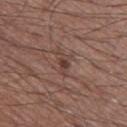This lesion was catalogued during total-body skin photography and was not selected for biopsy.
The lesion is on the leg.
Measured at roughly 3.5 mm in maximum diameter.
Automated tile analysis of the lesion measured a footprint of about 4 mm² and a symmetry-axis asymmetry near 0.4. It also reported a nevus-likeness score of about 0/100 and a detector confidence of about 100 out of 100 that the crop contains a lesion.
The patient is a male approximately 60 years of age.
A lesion tile, about 15 mm wide, cut from a 3D total-body photograph.